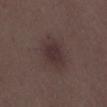Part of a total-body skin-imaging series; this lesion was reviewed on a skin check and was not flagged for biopsy. A lesion tile, about 15 mm wide, cut from a 3D total-body photograph. A male subject, aged 48–52.